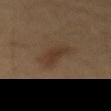A close-up tile cropped from a whole-body skin photograph, about 15 mm across. A male subject, aged 58 to 62. About 5 mm across. The lesion is located on the abdomen. Captured under cross-polarized illumination.